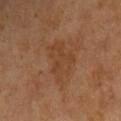Assessment:
Imaged during a routine full-body skin examination; the lesion was not biopsied and no histopathology is available.
Image and clinical context:
The subject is a male aged approximately 65. A close-up tile cropped from a whole-body skin photograph, about 15 mm across. The tile uses cross-polarized illumination. From the left arm.